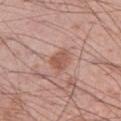| field | value |
|---|---|
| notes | catalogued during a skin exam; not biopsied |